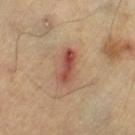follow-up = catalogued during a skin exam; not biopsied.